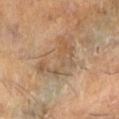The lesion was photographed on a routine skin check and not biopsied; there is no pathology result.
A roughly 15 mm field-of-view crop from a total-body skin photograph.
The tile uses cross-polarized illumination.
A male subject, approximately 60 years of age.
The lesion is on the leg.
Measured at roughly 6 mm in maximum diameter.